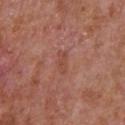Assessment:
Part of a total-body skin-imaging series; this lesion was reviewed on a skin check and was not flagged for biopsy.
Image and clinical context:
The patient is a male in their mid- to late 60s. A region of skin cropped from a whole-body photographic capture, roughly 15 mm wide. From the chest.